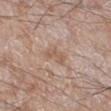The recorded lesion diameter is about 3 mm. The subject is a male roughly 60 years of age. Automated tile analysis of the lesion measured border irregularity of about 4 on a 0–10 scale, internal color variation of about 1 on a 0–10 scale, and radial color variation of about 0.5. And it measured a classifier nevus-likeness of about 0/100 and a detector confidence of about 100 out of 100 that the crop contains a lesion. On the right lower leg. A 15 mm crop from a total-body photograph taken for skin-cancer surveillance.